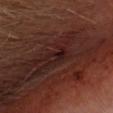Findings:
• workup — catalogued during a skin exam; not biopsied
• image-analysis metrics — a color-variation rating of about 5.5/10 and radial color variation of about 2
• subject — male, about 65 years old
• diameter — about 2.5 mm
• image — total-body-photography crop, ~15 mm field of view
• illumination — cross-polarized